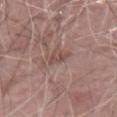workup: no biopsy performed (imaged during a skin exam)
lighting: white-light illumination
patient: male, aged approximately 70
anatomic site: the arm
TBP lesion metrics: an outline eccentricity of about 0.8 (0 = round, 1 = elongated) and two-axis asymmetry of about 0.35; a border-irregularity index near 4.5/10, internal color variation of about 0.5 on a 0–10 scale, and a peripheral color-asymmetry measure near 0; an automated nevus-likeness rating near 0 out of 100 and a detector confidence of about 80 out of 100 that the crop contains a lesion
size: about 2.5 mm
imaging modality: 15 mm crop, total-body photography